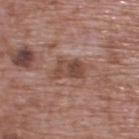– biopsy status — total-body-photography surveillance lesion; no biopsy
– subject — male, in their 70s
– tile lighting — white-light illumination
– size — about 4 mm
– image — ~15 mm tile from a whole-body skin photo
– location — the upper back
– automated metrics — a border-irregularity index near 3.5/10 and a peripheral color-asymmetry measure near 2; an automated nevus-likeness rating near 0 out of 100 and a detector confidence of about 100 out of 100 that the crop contains a lesion